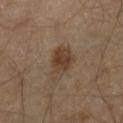biopsy status: imaged on a skin check; not biopsied
body site: the right lower leg
acquisition: ~15 mm crop, total-body skin-cancer survey
patient: male, in their mid- to late 40s